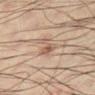{
  "biopsy_status": "not biopsied; imaged during a skin examination",
  "site": "left lower leg",
  "lighting": "cross-polarized",
  "patient": {
    "sex": "male",
    "age_approx": 35
  },
  "image": {
    "source": "total-body photography crop",
    "field_of_view_mm": 15
  }
}